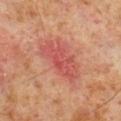<lesion>
<biopsy_status>not biopsied; imaged during a skin examination</biopsy_status>
<site>right lower leg</site>
<lesion_size>
  <long_diameter_mm_approx>5.5</long_diameter_mm_approx>
</lesion_size>
<patient>
  <sex>male</sex>
  <age_approx>60</age_approx>
</patient>
<image>
  <source>total-body photography crop</source>
  <field_of_view_mm>15</field_of_view_mm>
</image>
</lesion>